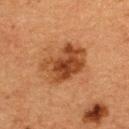<lesion>
  <lighting>cross-polarized</lighting>
  <automated_metrics>
    <vs_skin_darker_L>11.0</vs_skin_darker_L>
    <vs_skin_contrast_norm>9.0</vs_skin_contrast_norm>
  </automated_metrics>
  <site>upper back</site>
  <patient>
    <sex>female</sex>
    <age_approx>40</age_approx>
  </patient>
  <image>
    <source>total-body photography crop</source>
    <field_of_view_mm>15</field_of_view_mm>
  </image>
  <lesion_size>
    <long_diameter_mm_approx>5.5</long_diameter_mm_approx>
  </lesion_size>
</lesion>